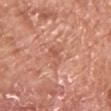No biopsy was performed on this lesion — it was imaged during a full skin examination and was not determined to be concerning.